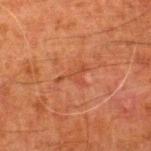The lesion is located on the right lower leg.
A male patient roughly 80 years of age.
A region of skin cropped from a whole-body photographic capture, roughly 15 mm wide.
The lesion-visualizer software estimated a lesion area of about 4 mm², an outline eccentricity of about 0.85 (0 = round, 1 = elongated), and a symmetry-axis asymmetry near 0.8.